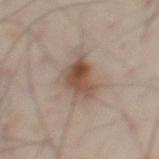Impression: Captured during whole-body skin photography for melanoma surveillance; the lesion was not biopsied. Background: A 15 mm crop from a total-body photograph taken for skin-cancer surveillance. From the abdomen. The lesion-visualizer software estimated a mean CIELAB color near L≈50 a*≈16 b*≈26 and about 12 CIELAB-L* units darker than the surrounding skin. The analysis additionally found an automated nevus-likeness rating near 90 out of 100 and a detector confidence of about 100 out of 100 that the crop contains a lesion. The recorded lesion diameter is about 5.5 mm. This is a cross-polarized tile. A male patient, in their mid- to late 50s.